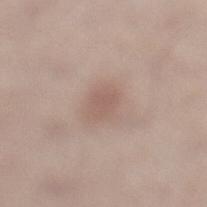Impression: Recorded during total-body skin imaging; not selected for excision or biopsy.